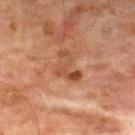Part of a total-body skin-imaging series; this lesion was reviewed on a skin check and was not flagged for biopsy. From the chest. A male subject aged 63–67. Cropped from a total-body skin-imaging series; the visible field is about 15 mm.